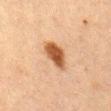notes: catalogued during a skin exam; not biopsied | subject: female, roughly 60 years of age | image source: ~15 mm tile from a whole-body skin photo | diameter: ≈4 mm | location: the abdomen.